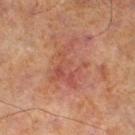notes = total-body-photography surveillance lesion; no biopsy.